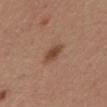Image and clinical context:
The lesion-visualizer software estimated an eccentricity of roughly 0.7 and a shape-asymmetry score of about 0.15 (0 = symmetric). The analysis additionally found an automated nevus-likeness rating near 95 out of 100 and a lesion-detection confidence of about 100/100. The subject is a female in their mid-60s. The lesion is located on the front of the torso. A 15 mm close-up extracted from a 3D total-body photography capture.